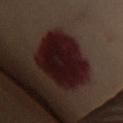Clinical impression:
The lesion was photographed on a routine skin check and not biopsied; there is no pathology result.
Image and clinical context:
The total-body-photography lesion software estimated a border-irregularity index near 1.5/10, a color-variation rating of about 4.5/10, and a peripheral color-asymmetry measure near 1.5. The software also gave a classifier nevus-likeness of about 35/100 and lesion-presence confidence of about 90/100. Approximately 7.5 mm at its widest. A female subject about 60 years old. From the chest. A region of skin cropped from a whole-body photographic capture, roughly 15 mm wide.